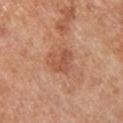  biopsy_status: not biopsied; imaged during a skin examination
  image:
    source: total-body photography crop
    field_of_view_mm: 15
  site: chest
  patient:
    sex: male
    age_approx: 30
  lesion_size:
    long_diameter_mm_approx: 3.0
  automated_metrics:
    area_mm2_approx: 6.0
    eccentricity: 0.5
    shape_asymmetry: 0.4